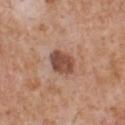The lesion was photographed on a routine skin check and not biopsied; there is no pathology result.
The recorded lesion diameter is about 3.5 mm.
Captured under white-light illumination.
A male patient, aged 63–67.
A roughly 15 mm field-of-view crop from a total-body skin photograph.
An algorithmic analysis of the crop reported a border-irregularity index near 1.5/10 and a peripheral color-asymmetry measure near 1. And it measured a nevus-likeness score of about 25/100 and a detector confidence of about 100 out of 100 that the crop contains a lesion.
The lesion is on the chest.